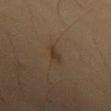<record>
  <automated_metrics>
    <cielab_L>33</cielab_L>
    <cielab_a>14</cielab_a>
    <cielab_b>26</cielab_b>
    <vs_skin_darker_L>6.0</vs_skin_darker_L>
    <vs_skin_contrast_norm>7.0</vs_skin_contrast_norm>
    <border_irregularity_0_10>3.5</border_irregularity_0_10>
    <color_variation_0_10>0.5</color_variation_0_10>
    <peripheral_color_asymmetry>0.0</peripheral_color_asymmetry>
    <lesion_detection_confidence_0_100>100</lesion_detection_confidence_0_100>
  </automated_metrics>
  <site>chest</site>
  <lighting>cross-polarized</lighting>
  <lesion_size>
    <long_diameter_mm_approx>2.5</long_diameter_mm_approx>
  </lesion_size>
  <image>
    <source>total-body photography crop</source>
    <field_of_view_mm>15</field_of_view_mm>
  </image>
  <patient>
    <sex>male</sex>
    <age_approx>55</age_approx>
  </patient>
</record>